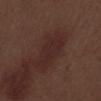<record>
  <site>left lower leg</site>
  <patient>
    <sex>male</sex>
    <age_approx>70</age_approx>
  </patient>
  <image>
    <source>total-body photography crop</source>
    <field_of_view_mm>15</field_of_view_mm>
  </image>
</record>